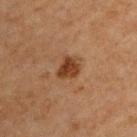biopsy status: total-body-photography surveillance lesion; no biopsy | body site: the arm | subject: male, aged around 70 | acquisition: 15 mm crop, total-body photography | diameter: ~3 mm (longest diameter).